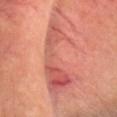Clinical impression: This lesion was catalogued during total-body skin photography and was not selected for biopsy. Acquisition and patient details: A female patient, aged 48–52. Imaged with cross-polarized lighting. About 10.5 mm across. The total-body-photography lesion software estimated an eccentricity of roughly 0.9 and a symmetry-axis asymmetry near 0.5. The analysis additionally found a border-irregularity index near 9/10, internal color variation of about 8 on a 0–10 scale, and peripheral color asymmetry of about 2.5. The software also gave a classifier nevus-likeness of about 0/100 and a detector confidence of about 70 out of 100 that the crop contains a lesion. Located on the head or neck. A region of skin cropped from a whole-body photographic capture, roughly 15 mm wide.